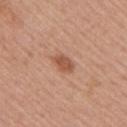The lesion was photographed on a routine skin check and not biopsied; there is no pathology result. From the right upper arm. The tile uses white-light illumination. A 15 mm crop from a total-body photograph taken for skin-cancer surveillance. About 3 mm across. A female subject aged 53 to 57. An algorithmic analysis of the crop reported an area of roughly 4.5 mm², an outline eccentricity of about 0.8 (0 = round, 1 = elongated), and a shape-asymmetry score of about 0.2 (0 = symmetric). And it measured a border-irregularity rating of about 2/10, a within-lesion color-variation index near 2/10, and radial color variation of about 0.5.